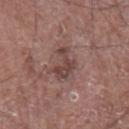diameter=~3.5 mm (longest diameter)
acquisition=total-body-photography crop, ~15 mm field of view
site=the left forearm
subject=male, in their mid-70s